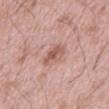patient:
  sex: male
  age_approx: 60
lighting: white-light
automated_metrics:
  area_mm2_approx: 5.0
  eccentricity: 0.7
  shape_asymmetry: 0.3
  cielab_L: 57
  cielab_a: 23
  cielab_b: 26
  vs_skin_contrast_norm: 7.0
site: front of the torso
image:
  source: total-body photography crop
  field_of_view_mm: 15
lesion_size:
  long_diameter_mm_approx: 3.0